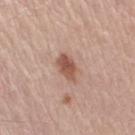The lesion was photographed on a routine skin check and not biopsied; there is no pathology result. A 15 mm close-up tile from a total-body photography series done for melanoma screening. Located on the arm. The recorded lesion diameter is about 3.5 mm. Captured under white-light illumination. A male subject, aged around 55.